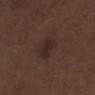notes: total-body-photography surveillance lesion; no biopsy | lighting: white-light illumination | body site: the right lower leg | subject: male, aged 68–72 | image source: 15 mm crop, total-body photography | size: about 3 mm.